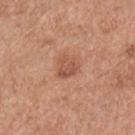workup: no biopsy performed (imaged during a skin exam) | tile lighting: white-light illumination | site: the chest | acquisition: ~15 mm tile from a whole-body skin photo | patient: male, approximately 55 years of age | diameter: about 2.5 mm | automated lesion analysis: a footprint of about 6 mm², an eccentricity of roughly 0.3, and a shape-asymmetry score of about 0.3 (0 = symmetric); border irregularity of about 2.5 on a 0–10 scale, a color-variation rating of about 3/10, and peripheral color asymmetry of about 1.